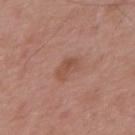No biopsy was performed on this lesion — it was imaged during a full skin examination and was not determined to be concerning. Imaged with white-light lighting. Located on the upper back. Approximately 3 mm at its widest. The subject is a male in their mid- to late 50s. A roughly 15 mm field-of-view crop from a total-body skin photograph.